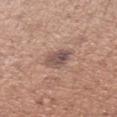Recorded during total-body skin imaging; not selected for excision or biopsy. A close-up tile cropped from a whole-body skin photograph, about 15 mm across. The subject is a female about 30 years old. The tile uses white-light illumination. Located on the left forearm.